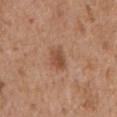Findings:
– workup: catalogued during a skin exam; not biopsied
– tile lighting: white-light illumination
– patient: male, about 65 years old
– imaging modality: ~15 mm tile from a whole-body skin photo
– lesion diameter: about 3 mm
– location: the left upper arm
– image-analysis metrics: a lesion area of about 5 mm², a shape eccentricity near 0.65, and a shape-asymmetry score of about 0.25 (0 = symmetric); a lesion color around L≈49 a*≈22 b*≈32 in CIELAB and roughly 10 lightness units darker than nearby skin; a border-irregularity index near 2.5/10, a color-variation rating of about 2.5/10, and peripheral color asymmetry of about 1